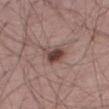Q: Was a biopsy performed?
A: total-body-photography surveillance lesion; no biopsy
Q: What kind of image is this?
A: ~15 mm tile from a whole-body skin photo
Q: What are the patient's age and sex?
A: male, aged 53 to 57
Q: Lesion location?
A: the leg